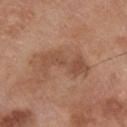| feature | finding |
|---|---|
| biopsy status | no biopsy performed (imaged during a skin exam) |
| lesion diameter | about 6 mm |
| location | the front of the torso |
| tile lighting | white-light |
| image source | 15 mm crop, total-body photography |
| subject | male, aged approximately 80 |